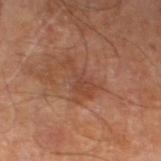Clinical impression:
Captured during whole-body skin photography for melanoma surveillance; the lesion was not biopsied.
Background:
The lesion is on the leg. The total-body-photography lesion software estimated a lesion color around L≈42 a*≈23 b*≈29 in CIELAB, a lesion–skin lightness drop of about 6, and a lesion-to-skin contrast of about 5 (normalized; higher = more distinct). And it measured a classifier nevus-likeness of about 0/100. A male subject approximately 60 years of age. A 15 mm close-up extracted from a 3D total-body photography capture.